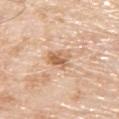{"biopsy_status": "not biopsied; imaged during a skin examination", "site": "upper back", "image": {"source": "total-body photography crop", "field_of_view_mm": 15}, "lighting": "white-light", "patient": {"sex": "male", "age_approx": 80}, "lesion_size": {"long_diameter_mm_approx": 3.5}}